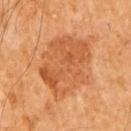The lesion was tiled from a total-body skin photograph and was not biopsied. The total-body-photography lesion software estimated a mean CIELAB color near L≈54 a*≈26 b*≈40, about 9 CIELAB-L* units darker than the surrounding skin, and a normalized lesion–skin contrast near 6.5. It also reported a classifier nevus-likeness of about 70/100 and a detector confidence of about 100 out of 100 that the crop contains a lesion. The recorded lesion diameter is about 7.5 mm. The tile uses cross-polarized illumination. On the right upper arm. The patient is a male in their 60s. This image is a 15 mm lesion crop taken from a total-body photograph.